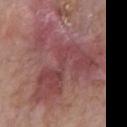follow-up: total-body-photography surveillance lesion; no biopsy